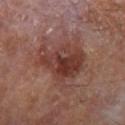Q: Was this lesion biopsied?
A: imaged on a skin check; not biopsied
Q: Lesion location?
A: the left forearm
Q: How was this image acquired?
A: 15 mm crop, total-body photography
Q: Automated lesion metrics?
A: a footprint of about 20 mm², an eccentricity of roughly 0.75, and two-axis asymmetry of about 0.35; border irregularity of about 4.5 on a 0–10 scale, a within-lesion color-variation index near 6/10, and radial color variation of about 2; a detector confidence of about 100 out of 100 that the crop contains a lesion
Q: What is the lesion's diameter?
A: ~6.5 mm (longest diameter)
Q: What are the patient's age and sex?
A: male, aged approximately 65